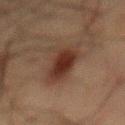biopsy status: no biopsy performed (imaged during a skin exam) | subject: male, about 60 years old | automated metrics: a lesion area of about 15 mm² and an outline eccentricity of about 0.9 (0 = round, 1 = elongated); a lesion color around L≈26 a*≈15 b*≈21 in CIELAB and a normalized border contrast of about 9; border irregularity of about 5.5 on a 0–10 scale and a within-lesion color-variation index near 4/10; a detector confidence of about 100 out of 100 that the crop contains a lesion | imaging modality: ~15 mm tile from a whole-body skin photo | illumination: cross-polarized | body site: the mid back.